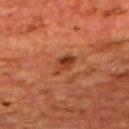The lesion was tiled from a total-body skin photograph and was not biopsied. The lesion's longest dimension is about 3.5 mm. On the upper back. A male patient aged 63 to 67. A region of skin cropped from a whole-body photographic capture, roughly 15 mm wide.